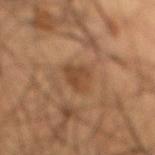Clinical impression:
The lesion was tiled from a total-body skin photograph and was not biopsied.
Background:
This image is a 15 mm lesion crop taken from a total-body photograph. The patient is a male roughly 55 years of age. The lesion is located on the front of the torso. The lesion's longest dimension is about 3 mm.